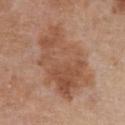biopsy_status: not biopsied; imaged during a skin examination
image:
  source: total-body photography crop
  field_of_view_mm: 15
patient:
  sex: female
  age_approx: 75
lighting: white-light
automated_metrics:
  cielab_L: 52
  cielab_a: 21
  cielab_b: 31
  vs_skin_darker_L: 9.0
  border_irregularity_0_10: 3.5
  color_variation_0_10: 5.5
  peripheral_color_asymmetry: 2.0
site: front of the torso
lesion_size:
  long_diameter_mm_approx: 10.0